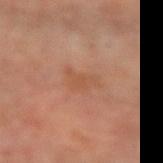This lesion was catalogued during total-body skin photography and was not selected for biopsy. Located on the right forearm. Measured at roughly 3.5 mm in maximum diameter. Imaged with cross-polarized lighting. The total-body-photography lesion software estimated a lesion area of about 6 mm², an eccentricity of roughly 0.75, and a shape-asymmetry score of about 0.45 (0 = symmetric). And it measured border irregularity of about 5 on a 0–10 scale and radial color variation of about 0.5. The analysis additionally found lesion-presence confidence of about 100/100. A female subject aged 28 to 32. A roughly 15 mm field-of-view crop from a total-body skin photograph.